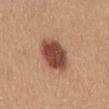  biopsy_status: not biopsied; imaged during a skin examination
  lighting: white-light
  image:
    source: total-body photography crop
    field_of_view_mm: 15
  lesion_size:
    long_diameter_mm_approx: 5.0
  automated_metrics:
    area_mm2_approx: 13.0
    eccentricity: 0.7
    lesion_detection_confidence_0_100: 100
  patient:
    sex: female
    age_approx: 55
  site: abdomen